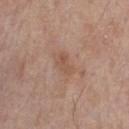Assessment: Imaged during a routine full-body skin examination; the lesion was not biopsied and no histopathology is available. Clinical summary: A 15 mm crop from a total-body photograph taken for skin-cancer surveillance. A male subject, aged 78 to 82. From the front of the torso. This is a white-light tile.